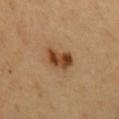• notes · imaged on a skin check; not biopsied
• acquisition · ~15 mm tile from a whole-body skin photo
• image-analysis metrics · a shape eccentricity near 0.75 and a shape-asymmetry score of about 0.25 (0 = symmetric); a mean CIELAB color near L≈43 a*≈20 b*≈35; a border-irregularity index near 2.5/10, internal color variation of about 5.5 on a 0–10 scale, and a peripheral color-asymmetry measure near 2; an automated nevus-likeness rating near 100 out of 100 and a lesion-detection confidence of about 100/100
• site · the front of the torso
• lesion diameter · ≈3.5 mm
• lighting · cross-polarized illumination
• subject · male, approximately 60 years of age